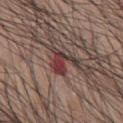The lesion was photographed on a routine skin check and not biopsied; there is no pathology result.